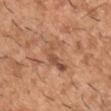Assessment:
The lesion was tiled from a total-body skin photograph and was not biopsied.
Acquisition and patient details:
Measured at roughly 4.5 mm in maximum diameter. Imaged with white-light lighting. The patient is a male in their 40s. A 15 mm crop from a total-body photograph taken for skin-cancer surveillance. The lesion is on the left upper arm.